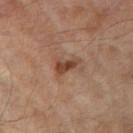| key | value |
|---|---|
| follow-up | total-body-photography surveillance lesion; no biopsy |